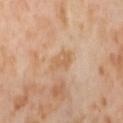Clinical summary:
A roughly 15 mm field-of-view crop from a total-body skin photograph. Imaged with cross-polarized lighting. A female subject, aged 53 to 57. The lesion is located on the right thigh. The recorded lesion diameter is about 3 mm.